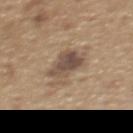- workup: no biopsy performed (imaged during a skin exam)
- patient: male, aged 63 to 67
- site: the upper back
- imaging modality: ~15 mm tile from a whole-body skin photo
- automated metrics: an area of roughly 12 mm², a shape eccentricity near 0.5, and a symmetry-axis asymmetry near 0.35; a lesion color around L≈50 a*≈14 b*≈26 in CIELAB; a border-irregularity rating of about 4.5/10, internal color variation of about 6.5 on a 0–10 scale, and a peripheral color-asymmetry measure near 2.5; a classifier nevus-likeness of about 25/100
- tile lighting: white-light